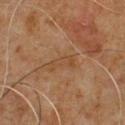No biopsy was performed on this lesion — it was imaged during a full skin examination and was not determined to be concerning.
A close-up tile cropped from a whole-body skin photograph, about 15 mm across.
This is a cross-polarized tile.
About 3.5 mm across.
A male subject approximately 60 years of age.
The lesion is located on the front of the torso.